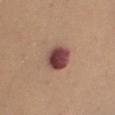Imaged during a routine full-body skin examination; the lesion was not biopsied and no histopathology is available.
On the right upper arm.
This image is a 15 mm lesion crop taken from a total-body photograph.
A male subject aged around 40.